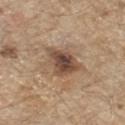Impression:
No biopsy was performed on this lesion — it was imaged during a full skin examination and was not determined to be concerning.
Clinical summary:
Captured under white-light illumination. The patient is a male aged approximately 70. From the front of the torso. About 5 mm across. Cropped from a total-body skin-imaging series; the visible field is about 15 mm.